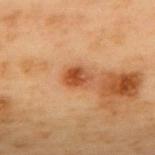Q: Is there a histopathology result?
A: total-body-photography surveillance lesion; no biopsy
Q: What kind of image is this?
A: ~15 mm tile from a whole-body skin photo
Q: Illumination type?
A: cross-polarized
Q: Where on the body is the lesion?
A: the upper back
Q: What are the patient's age and sex?
A: male, roughly 55 years of age